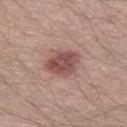| key | value |
|---|---|
| lesion diameter | ≈4 mm |
| subject | male, aged approximately 40 |
| site | the left thigh |
| image-analysis metrics | a lesion area of about 9.5 mm², an outline eccentricity of about 0.6 (0 = round, 1 = elongated), and a shape-asymmetry score of about 0.25 (0 = symmetric); a nevus-likeness score of about 90/100 and a lesion-detection confidence of about 100/100 |
| tile lighting | white-light |
| image | ~15 mm tile from a whole-body skin photo |